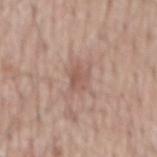<tbp_lesion>
  <biopsy_status>not biopsied; imaged during a skin examination</biopsy_status>
  <lighting>white-light</lighting>
  <automated_metrics>
    <area_mm2_approx>4.0</area_mm2_approx>
    <eccentricity>0.75</eccentricity>
  </automated_metrics>
  <image>
    <source>total-body photography crop</source>
    <field_of_view_mm>15</field_of_view_mm>
  </image>
  <lesion_size>
    <long_diameter_mm_approx>3.0</long_diameter_mm_approx>
  </lesion_size>
  <patient>
    <sex>male</sex>
    <age_approx>65</age_approx>
  </patient>
  <site>mid back</site>
</tbp_lesion>